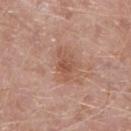workup — catalogued during a skin exam; not biopsied
anatomic site — the right lower leg
imaging modality — 15 mm crop, total-body photography
patient — male, roughly 50 years of age